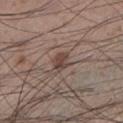Clinical impression: The lesion was tiled from a total-body skin photograph and was not biopsied. Background: A roughly 15 mm field-of-view crop from a total-body skin photograph. A male subject, aged around 35. This is a white-light tile. From the left lower leg. The recorded lesion diameter is about 2.5 mm.